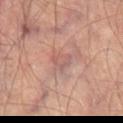• notes: total-body-photography surveillance lesion; no biopsy
• automated metrics: a footprint of about 4.5 mm²; an average lesion color of about L≈55 a*≈21 b*≈23 (CIELAB), roughly 6 lightness units darker than nearby skin, and a normalized border contrast of about 5; a border-irregularity index near 3/10, a within-lesion color-variation index near 2.5/10, and radial color variation of about 1
• image: ~15 mm tile from a whole-body skin photo
• patient: male, about 70 years old
• site: the left thigh